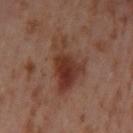Imaged during a routine full-body skin examination; the lesion was not biopsied and no histopathology is available.
The total-body-photography lesion software estimated an area of roughly 17 mm², an eccentricity of roughly 0.85, and two-axis asymmetry of about 0.45. And it measured a mean CIELAB color near L≈37 a*≈22 b*≈26, about 10 CIELAB-L* units darker than the surrounding skin, and a normalized lesion–skin contrast near 8.5. It also reported a border-irregularity rating of about 6/10, a within-lesion color-variation index near 8/10, and radial color variation of about 2.5. The analysis additionally found a classifier nevus-likeness of about 70/100 and a detector confidence of about 100 out of 100 that the crop contains a lesion.
Measured at roughly 7 mm in maximum diameter.
The subject is a female in their mid-50s.
Cropped from a whole-body photographic skin survey; the tile spans about 15 mm.
The tile uses cross-polarized illumination.
On the left thigh.